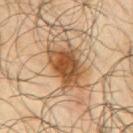biopsy_status: not biopsied; imaged during a skin examination
image:
  source: total-body photography crop
  field_of_view_mm: 15
patient:
  sex: male
  age_approx: 65
lesion_size:
  long_diameter_mm_approx: 6.0
site: arm
lighting: cross-polarized
automated_metrics:
  area_mm2_approx: 14.0
  eccentricity: 0.8
  shape_asymmetry: 0.3